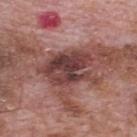follow-up=catalogued during a skin exam; not biopsied | patient=male, approximately 70 years of age | automated metrics=an area of roughly 18 mm² and a shape eccentricity near 0.75; a peripheral color-asymmetry measure near 2.5; an automated nevus-likeness rating near 0 out of 100 and lesion-presence confidence of about 95/100 | image source=~15 mm crop, total-body skin-cancer survey | site=the mid back | diameter=~6.5 mm (longest diameter).